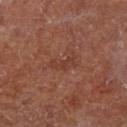| feature | finding |
|---|---|
| notes | imaged on a skin check; not biopsied |
| acquisition | 15 mm crop, total-body photography |
| anatomic site | the leg |
| lighting | cross-polarized illumination |
| subject | female |
| size | about 3.5 mm |